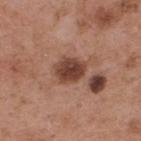Recorded during total-body skin imaging; not selected for excision or biopsy.
A 15 mm close-up extracted from a 3D total-body photography capture.
Located on the upper back.
The subject is a male about 55 years old.